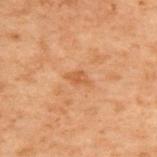The lesion was photographed on a routine skin check and not biopsied; there is no pathology result. A 15 mm close-up extracted from a 3D total-body photography capture. The lesion-visualizer software estimated an area of roughly 3 mm², an eccentricity of roughly 0.8, and two-axis asymmetry of about 0.3. The software also gave a lesion–skin lightness drop of about 6 and a normalized lesion–skin contrast near 5.5. It also reported a border-irregularity index near 2.5/10, a within-lesion color-variation index near 1.5/10, and peripheral color asymmetry of about 0.5. A male patient, aged 68 to 72. Imaged with cross-polarized lighting. The lesion's longest dimension is about 2.5 mm. From the upper back.